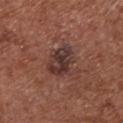Q: Is there a histopathology result?
A: imaged on a skin check; not biopsied